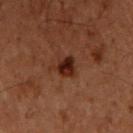| key | value |
|---|---|
| workup | imaged on a skin check; not biopsied |
| size | ~2.5 mm (longest diameter) |
| automated lesion analysis | a lesion area of about 5 mm², a shape eccentricity near 0.6, and a symmetry-axis asymmetry near 0.25; a normalized lesion–skin contrast near 11.5; a border-irregularity rating of about 2.5/10 and a peripheral color-asymmetry measure near 1; lesion-presence confidence of about 100/100 |
| site | the front of the torso |
| subject | male, roughly 60 years of age |
| illumination | cross-polarized illumination |
| image source | ~15 mm crop, total-body skin-cancer survey |